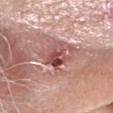Findings:
* follow-up · catalogued during a skin exam; not biopsied
* acquisition · ~15 mm tile from a whole-body skin photo
* patient · male, about 65 years old
* diameter · ~3.5 mm (longest diameter)
* anatomic site · the head or neck